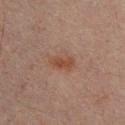Impression: No biopsy was performed on this lesion — it was imaged during a full skin examination and was not determined to be concerning. Context: The lesion's longest dimension is about 3 mm. A male patient aged approximately 30. A lesion tile, about 15 mm wide, cut from a 3D total-body photograph. An algorithmic analysis of the crop reported a lesion area of about 5 mm² and a symmetry-axis asymmetry near 0.25. The software also gave a lesion color around L≈38 a*≈17 b*≈25 in CIELAB and about 6 CIELAB-L* units darker than the surrounding skin. And it measured border irregularity of about 3 on a 0–10 scale, internal color variation of about 2 on a 0–10 scale, and radial color variation of about 0.5. It also reported a classifier nevus-likeness of about 15/100. The lesion is on the chest. This is a cross-polarized tile.